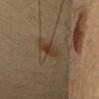follow-up=imaged on a skin check; not biopsied
location=the head or neck
subject=female, in their mid-30s
lesion size=~2.5 mm (longest diameter)
image=15 mm crop, total-body photography
automated metrics=an area of roughly 4 mm², a shape eccentricity near 0.7, and a symmetry-axis asymmetry near 0.35; an automated nevus-likeness rating near 65 out of 100 and a detector confidence of about 100 out of 100 that the crop contains a lesion